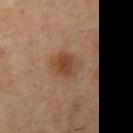<lesion>
  <patient>
    <sex>male</sex>
    <age_approx>55</age_approx>
  </patient>
  <image>
    <source>total-body photography crop</source>
    <field_of_view_mm>15</field_of_view_mm>
  </image>
  <site>left upper arm</site>
  <lighting>cross-polarized</lighting>
</lesion>